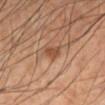This lesion was catalogued during total-body skin photography and was not selected for biopsy. About 2.5 mm across. A region of skin cropped from a whole-body photographic capture, roughly 15 mm wide. The lesion is on the left lower leg. Imaged with cross-polarized lighting. A male patient about 50 years old. Automated image analysis of the tile measured a lesion area of about 3.5 mm², an eccentricity of roughly 0.85, and a symmetry-axis asymmetry near 0.25. The software also gave a mean CIELAB color near L≈49 a*≈22 b*≈33, roughly 10 lightness units darker than nearby skin, and a normalized lesion–skin contrast near 7.